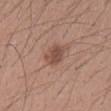Assessment: The lesion was tiled from a total-body skin photograph and was not biopsied. Clinical summary: An algorithmic analysis of the crop reported a lesion color around L≈49 a*≈21 b*≈26 in CIELAB, a lesion–skin lightness drop of about 10, and a lesion-to-skin contrast of about 7.5 (normalized; higher = more distinct). And it measured a detector confidence of about 100 out of 100 that the crop contains a lesion. A lesion tile, about 15 mm wide, cut from a 3D total-body photograph. A male subject aged 38–42. Located on the mid back. Measured at roughly 3.5 mm in maximum diameter.